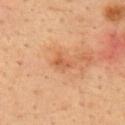This lesion was catalogued during total-body skin photography and was not selected for biopsy.
Cropped from a total-body skin-imaging series; the visible field is about 15 mm.
The lesion is located on the upper back.
A male subject, aged 33–37.